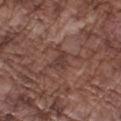Clinical impression:
Captured during whole-body skin photography for melanoma surveillance; the lesion was not biopsied.
Background:
The lesion is on the arm. The subject is a male aged approximately 75. The total-body-photography lesion software estimated an automated nevus-likeness rating near 0 out of 100 and lesion-presence confidence of about 95/100. The recorded lesion diameter is about 2.5 mm. A 15 mm close-up extracted from a 3D total-body photography capture. Captured under white-light illumination.